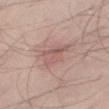Clinical impression: The lesion was photographed on a routine skin check and not biopsied; there is no pathology result. Background: A male patient about 35 years old. Captured under white-light illumination. A 15 mm close-up tile from a total-body photography series done for melanoma screening. The lesion is on the right thigh.